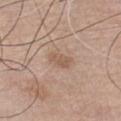workup: no biopsy performed (imaged during a skin exam)
image source: ~15 mm crop, total-body skin-cancer survey
lesion diameter: ≈3 mm
subject: male, aged 73–77
anatomic site: the chest
lighting: white-light illumination
automated metrics: a lesion area of about 4 mm², a shape eccentricity near 0.85, and a shape-asymmetry score of about 0.25 (0 = symmetric)